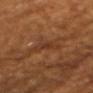The lesion was tiled from a total-body skin photograph and was not biopsied.
From the head or neck.
Measured at roughly 4 mm in maximum diameter.
This is a cross-polarized tile.
A 15 mm close-up extracted from a 3D total-body photography capture.
The patient is a male aged around 60.
Automated tile analysis of the lesion measured an outline eccentricity of about 0.95 (0 = round, 1 = elongated) and two-axis asymmetry of about 0.4. The analysis additionally found border irregularity of about 5 on a 0–10 scale, a color-variation rating of about 1/10, and peripheral color asymmetry of about 0.5. The software also gave an automated nevus-likeness rating near 0 out of 100 and a detector confidence of about 55 out of 100 that the crop contains a lesion.